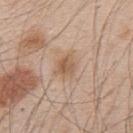Recorded during total-body skin imaging; not selected for excision or biopsy. The lesion is located on the back. This is a white-light tile. An algorithmic analysis of the crop reported a lesion area of about 5.5 mm² and a shape eccentricity near 0.5. The software also gave border irregularity of about 2.5 on a 0–10 scale, a color-variation rating of about 3/10, and a peripheral color-asymmetry measure near 1. A lesion tile, about 15 mm wide, cut from a 3D total-body photograph. The subject is a male approximately 50 years of age. Longest diameter approximately 3 mm.